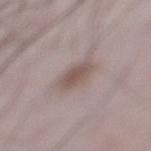workup: imaged on a skin check; not biopsied
location: the left lower leg
illumination: white-light illumination
size: ≈3.5 mm
acquisition: ~15 mm tile from a whole-body skin photo
TBP lesion metrics: an area of roughly 7 mm², a shape eccentricity near 0.55, and a shape-asymmetry score of about 0.15 (0 = symmetric); a nevus-likeness score of about 75/100
subject: male, about 50 years old